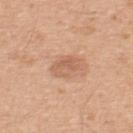biopsy_status: not biopsied; imaged during a skin examination
lighting: white-light
patient:
  sex: male
  age_approx: 50
lesion_size:
  long_diameter_mm_approx: 3.5
automated_metrics:
  area_mm2_approx: 6.5
  eccentricity: 0.7
  shape_asymmetry: 0.25
  border_irregularity_0_10: 2.5
  color_variation_0_10: 3.5
  peripheral_color_asymmetry: 1.0
site: upper back
image:
  source: total-body photography crop
  field_of_view_mm: 15